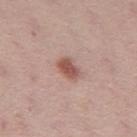Notes:
• biopsy status · imaged on a skin check; not biopsied
• tile lighting · white-light illumination
• image source · 15 mm crop, total-body photography
• lesion size · about 3 mm
• site · the leg
• patient · female, aged 28–32